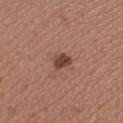Captured during whole-body skin photography for melanoma surveillance; the lesion was not biopsied.
The tile uses white-light illumination.
The lesion's longest dimension is about 2.5 mm.
The lesion is located on the right thigh.
A roughly 15 mm field-of-view crop from a total-body skin photograph.
The patient is a female aged approximately 30.